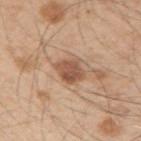Recorded during total-body skin imaging; not selected for excision or biopsy. The tile uses white-light illumination. Located on the right upper arm. The subject is a male in their 50s. An algorithmic analysis of the crop reported a footprint of about 6.5 mm² and an eccentricity of roughly 0.5. The software also gave a lesion color around L≈53 a*≈21 b*≈31 in CIELAB and about 12 CIELAB-L* units darker than the surrounding skin. And it measured lesion-presence confidence of about 100/100. Approximately 3 mm at its widest. A 15 mm crop from a total-body photograph taken for skin-cancer surveillance.